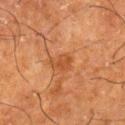Recorded during total-body skin imaging; not selected for excision or biopsy. This image is a 15 mm lesion crop taken from a total-body photograph. This is a cross-polarized tile. A male subject aged 63–67. The lesion-visualizer software estimated a lesion area of about 4.5 mm². The analysis additionally found a lesion color around L≈41 a*≈24 b*≈34 in CIELAB, about 7 CIELAB-L* units darker than the surrounding skin, and a normalized lesion–skin contrast near 6. The software also gave border irregularity of about 3.5 on a 0–10 scale and radial color variation of about 0.5. And it measured an automated nevus-likeness rating near 5 out of 100 and a lesion-detection confidence of about 100/100. From the right lower leg.